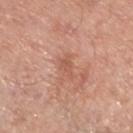Findings:
- follow-up: total-body-photography surveillance lesion; no biopsy
- body site: the left lower leg
- image: total-body-photography crop, ~15 mm field of view
- subject: male, approximately 50 years of age